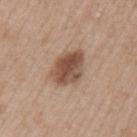Assessment:
The lesion was photographed on a routine skin check and not biopsied; there is no pathology result.
Context:
On the left upper arm. A close-up tile cropped from a whole-body skin photograph, about 15 mm across. A female patient about 40 years old. Measured at roughly 5 mm in maximum diameter. Captured under white-light illumination.